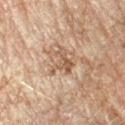Q: Was this lesion biopsied?
A: catalogued during a skin exam; not biopsied
Q: Where on the body is the lesion?
A: the right forearm
Q: What are the patient's age and sex?
A: male, in their mid-40s
Q: What kind of image is this?
A: ~15 mm crop, total-body skin-cancer survey
Q: What did automated image analysis measure?
A: a lesion area of about 5.5 mm², an outline eccentricity of about 0.8 (0 = round, 1 = elongated), and a shape-asymmetry score of about 0.55 (0 = symmetric); lesion-presence confidence of about 80/100
Q: How large is the lesion?
A: ~4 mm (longest diameter)